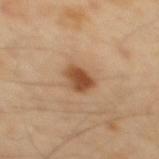biopsy_status: not biopsied; imaged during a skin examination
image:
  source: total-body photography crop
  field_of_view_mm: 15
site: mid back
patient:
  sex: male
  age_approx: 40
automated_metrics:
  cielab_L: 49
  cielab_a: 22
  cielab_b: 35
  vs_skin_darker_L: 14.0
  vs_skin_contrast_norm: 10.0
lesion_size:
  long_diameter_mm_approx: 3.0